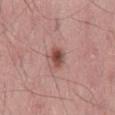Q: Was a biopsy performed?
A: catalogued during a skin exam; not biopsied
Q: What is the imaging modality?
A: total-body-photography crop, ~15 mm field of view
Q: Illumination type?
A: white-light illumination
Q: Lesion location?
A: the mid back
Q: Patient demographics?
A: male, roughly 60 years of age
Q: Lesion size?
A: about 3 mm